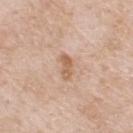Q: Was this lesion biopsied?
A: total-body-photography surveillance lesion; no biopsy
Q: Who is the patient?
A: male, aged approximately 70
Q: How large is the lesion?
A: about 3 mm
Q: What kind of image is this?
A: total-body-photography crop, ~15 mm field of view
Q: Where on the body is the lesion?
A: the upper back
Q: What did automated image analysis measure?
A: a footprint of about 3.5 mm², an eccentricity of roughly 0.9, and two-axis asymmetry of about 0.25; a mean CIELAB color near L≈61 a*≈20 b*≈33, roughly 10 lightness units darker than nearby skin, and a normalized lesion–skin contrast near 7.5; a border-irregularity index near 3/10, a color-variation rating of about 1/10, and a peripheral color-asymmetry measure near 0